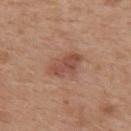notes: no biopsy performed (imaged during a skin exam) | site: the back | image-analysis metrics: a lesion color around L≈50 a*≈23 b*≈29 in CIELAB, about 9 CIELAB-L* units darker than the surrounding skin, and a normalized lesion–skin contrast near 7; an automated nevus-likeness rating near 20 out of 100 | patient: male, approximately 30 years of age | diameter: about 4.5 mm | image source: ~15 mm tile from a whole-body skin photo | illumination: white-light.